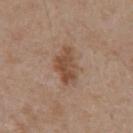Impression:
Part of a total-body skin-imaging series; this lesion was reviewed on a skin check and was not flagged for biopsy.
Acquisition and patient details:
A male patient, about 65 years old. Cropped from a whole-body photographic skin survey; the tile spans about 15 mm. An algorithmic analysis of the crop reported an area of roughly 8.5 mm², an eccentricity of roughly 0.85, and a symmetry-axis asymmetry near 0.25. The analysis additionally found an average lesion color of about L≈48 a*≈19 b*≈30 (CIELAB). And it measured a classifier nevus-likeness of about 5/100 and a detector confidence of about 100 out of 100 that the crop contains a lesion. The tile uses white-light illumination. Located on the chest. Measured at roughly 4.5 mm in maximum diameter.